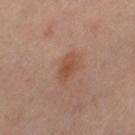This lesion was catalogued during total-body skin photography and was not selected for biopsy. A female patient approximately 50 years of age. On the right thigh. The lesion's longest dimension is about 3.5 mm. Captured under cross-polarized illumination. A close-up tile cropped from a whole-body skin photograph, about 15 mm across.